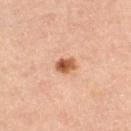{
  "biopsy_status": "not biopsied; imaged during a skin examination",
  "lesion_size": {
    "long_diameter_mm_approx": 2.5
  },
  "image": {
    "source": "total-body photography crop",
    "field_of_view_mm": 15
  },
  "automated_metrics": {
    "area_mm2_approx": 4.0,
    "eccentricity": 0.6,
    "cielab_L": 58,
    "cielab_a": 25,
    "cielab_b": 36,
    "vs_skin_darker_L": 16.0,
    "vs_skin_contrast_norm": 10.0,
    "border_irregularity_0_10": 1.5,
    "color_variation_0_10": 6.0,
    "nevus_likeness_0_100": 95
  },
  "site": "leg",
  "lighting": "cross-polarized",
  "patient": {
    "sex": "female",
    "age_approx": 55
  }
}